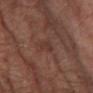follow-up: imaged on a skin check; not biopsied
subject: male, about 65 years old
imaging modality: ~15 mm tile from a whole-body skin photo
lesion size: about 3 mm
body site: the left thigh
lighting: cross-polarized